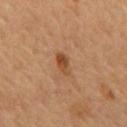| feature | finding |
|---|---|
| anatomic site | the back |
| automated lesion analysis | a footprint of about 4 mm² and a symmetry-axis asymmetry near 0.2; internal color variation of about 3.5 on a 0–10 scale and radial color variation of about 1.5; a classifier nevus-likeness of about 85/100 |
| image source | ~15 mm crop, total-body skin-cancer survey |
| lighting | cross-polarized |
| patient | male, in their mid- to late 50s |